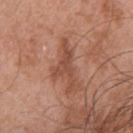Impression:
Imaged during a routine full-body skin examination; the lesion was not biopsied and no histopathology is available.
Context:
A male patient aged 68–72. Located on the chest. A roughly 15 mm field-of-view crop from a total-body skin photograph.